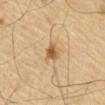notes — catalogued during a skin exam; not biopsied | image source — ~15 mm crop, total-body skin-cancer survey | patient — male, roughly 65 years of age | diameter — ≈3 mm | lighting — cross-polarized illumination.